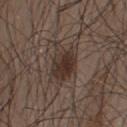Automated image analysis of the tile measured a lesion area of about 12 mm², an eccentricity of roughly 0.65, and a symmetry-axis asymmetry near 0.35. It also reported about 9 CIELAB-L* units darker than the surrounding skin and a normalized border contrast of about 8.5. It also reported a nevus-likeness score of about 95/100. From the upper back. This is a white-light tile. Approximately 4.5 mm at its widest. A 15 mm close-up extracted from a 3D total-body photography capture. The patient is a male about 50 years old.